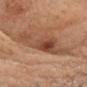Measured at roughly 8 mm in maximum diameter. This is a white-light tile. The subject is a male aged 68–72. A 15 mm crop from a total-body photograph taken for skin-cancer surveillance. The lesion is located on the head or neck.